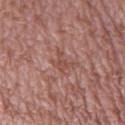  biopsy_status: not biopsied; imaged during a skin examination
  image:
    source: total-body photography crop
    field_of_view_mm: 15
  site: left lower leg
  patient:
    sex: male
    age_approx: 55
  automated_metrics:
    cielab_L: 49
    cielab_a: 23
    cielab_b: 26
    vs_skin_darker_L: 7.0
    vs_skin_contrast_norm: 5.5
  lighting: white-light